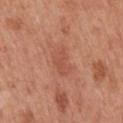Captured during whole-body skin photography for melanoma surveillance; the lesion was not biopsied.
A male patient, aged around 70.
The lesion's longest dimension is about 3.5 mm.
The tile uses white-light illumination.
The lesion is on the mid back.
Cropped from a whole-body photographic skin survey; the tile spans about 15 mm.